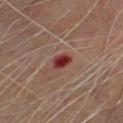Context:
The patient is a male aged around 65. The lesion is located on the chest. Cropped from a total-body skin-imaging series; the visible field is about 15 mm.